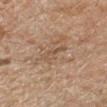  biopsy_status: not biopsied; imaged during a skin examination
  patient:
    sex: male
    age_approx: 45
  site: right forearm
  image:
    source: total-body photography crop
    field_of_view_mm: 15
  automated_metrics:
    eccentricity: 0.9
    shape_asymmetry: 0.4
    cielab_L: 40
    cielab_a: 13
    cielab_b: 24
    vs_skin_darker_L: 5.0
    vs_skin_contrast_norm: 4.5
    border_irregularity_0_10: 5.0
    color_variation_0_10: 0.0
    nevus_likeness_0_100: 0
    lesion_detection_confidence_0_100: 80
  lesion_size:
    long_diameter_mm_approx: 3.0
  lighting: cross-polarized